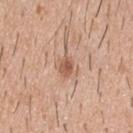No biopsy was performed on this lesion — it was imaged during a full skin examination and was not determined to be concerning.
A male patient, in their 40s.
Longest diameter approximately 2.5 mm.
This image is a 15 mm lesion crop taken from a total-body photograph.
From the upper back.